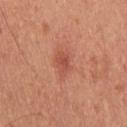Q: Was a biopsy performed?
A: catalogued during a skin exam; not biopsied
Q: How was the tile lit?
A: white-light
Q: What is the imaging modality?
A: 15 mm crop, total-body photography
Q: Where on the body is the lesion?
A: the upper back
Q: What are the patient's age and sex?
A: male, about 45 years old
Q: Automated lesion metrics?
A: an average lesion color of about L≈52 a*≈30 b*≈31 (CIELAB) and about 9 CIELAB-L* units darker than the surrounding skin; border irregularity of about 3.5 on a 0–10 scale, internal color variation of about 2 on a 0–10 scale, and a peripheral color-asymmetry measure near 0.5; a detector confidence of about 100 out of 100 that the crop contains a lesion